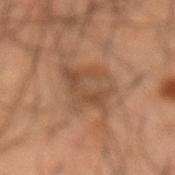Q: Is there a histopathology result?
A: imaged on a skin check; not biopsied
Q: What kind of image is this?
A: ~15 mm tile from a whole-body skin photo
Q: What is the anatomic site?
A: the abdomen
Q: What are the patient's age and sex?
A: male, roughly 55 years of age
Q: How large is the lesion?
A: ~5 mm (longest diameter)
Q: What lighting was used for the tile?
A: cross-polarized illumination